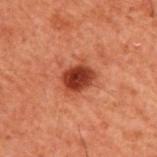Notes:
– biopsy status · catalogued during a skin exam; not biopsied